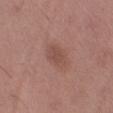{
  "biopsy_status": "not biopsied; imaged during a skin examination",
  "image": {
    "source": "total-body photography crop",
    "field_of_view_mm": 15
  },
  "automated_metrics": {
    "area_mm2_approx": 4.5,
    "eccentricity": 0.7,
    "shape_asymmetry": 0.25,
    "cielab_L": 48,
    "cielab_a": 21,
    "cielab_b": 24,
    "vs_skin_darker_L": 7.0,
    "vs_skin_contrast_norm": 5.5
  },
  "lesion_size": {
    "long_diameter_mm_approx": 3.0
  },
  "patient": {
    "sex": "male",
    "age_approx": 50
  },
  "site": "left thigh"
}